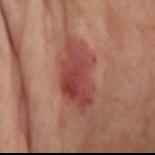Case summary:
– follow-up · imaged on a skin check; not biopsied
– image-analysis metrics · a shape eccentricity near 0.85 and two-axis asymmetry of about 0.3; an average lesion color of about L≈43 a*≈29 b*≈25 (CIELAB); a classifier nevus-likeness of about 80/100 and lesion-presence confidence of about 100/100
– location · the right forearm
– subject · female, in their mid-60s
– size · ~7 mm (longest diameter)
– lighting · cross-polarized
– imaging modality · 15 mm crop, total-body photography